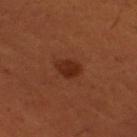Context:
The lesion is on the right thigh. Imaged with cross-polarized lighting. The subject is a male aged around 50. Approximately 3 mm at its widest. A 15 mm close-up tile from a total-body photography series done for melanoma screening.